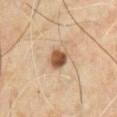Acquisition and patient details:
The total-body-photography lesion software estimated an outline eccentricity of about 0.5 (0 = round, 1 = elongated) and two-axis asymmetry of about 0.2. The software also gave an average lesion color of about L≈53 a*≈20 b*≈34 (CIELAB) and a lesion–skin lightness drop of about 17. The software also gave a classifier nevus-likeness of about 100/100 and lesion-presence confidence of about 100/100. Cropped from a whole-body photographic skin survey; the tile spans about 15 mm. Measured at roughly 2.5 mm in maximum diameter. From the chest. Captured under cross-polarized illumination. The subject is a male about 65 years old.